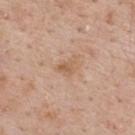- workup · no biopsy performed (imaged during a skin exam)
- tile lighting · white-light
- image source · ~15 mm crop, total-body skin-cancer survey
- subject · male, aged approximately 60
- location · the upper back
- automated metrics · a within-lesion color-variation index near 2.5/10 and peripheral color asymmetry of about 1; a nevus-likeness score of about 0/100 and a detector confidence of about 100 out of 100 that the crop contains a lesion
- size · about 2.5 mm